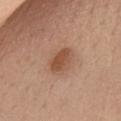{"biopsy_status": "not biopsied; imaged during a skin examination", "site": "front of the torso", "lesion_size": {"long_diameter_mm_approx": 3.5}, "patient": {"sex": "female", "age_approx": 40}, "lighting": "white-light", "image": {"source": "total-body photography crop", "field_of_view_mm": 15}}